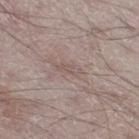Captured during whole-body skin photography for melanoma surveillance; the lesion was not biopsied. From the right thigh. The tile uses white-light illumination. Cropped from a whole-body photographic skin survey; the tile spans about 15 mm. A male subject about 50 years old. The lesion-visualizer software estimated an area of roughly 2.5 mm², an outline eccentricity of about 0.9 (0 = round, 1 = elongated), and a symmetry-axis asymmetry near 0.4. The analysis additionally found border irregularity of about 4 on a 0–10 scale and a within-lesion color-variation index near 0/10.